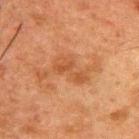Clinical impression:
Part of a total-body skin-imaging series; this lesion was reviewed on a skin check and was not flagged for biopsy.